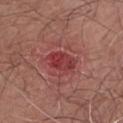| field | value |
|---|---|
| tile lighting | white-light illumination |
| image | ~15 mm crop, total-body skin-cancer survey |
| body site | the right forearm |
| automated metrics | an area of roughly 7 mm², an outline eccentricity of about 0.75 (0 = round, 1 = elongated), and two-axis asymmetry of about 0.2; a mean CIELAB color near L≈40 a*≈33 b*≈23 and a lesion–skin lightness drop of about 9; a border-irregularity index near 2.5/10, a within-lesion color-variation index near 5/10, and a peripheral color-asymmetry measure near 2; a classifier nevus-likeness of about 0/100 and lesion-presence confidence of about 100/100 |
| patient | male, approximately 55 years of age |